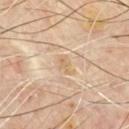A male patient aged 63–67. Located on the chest. Approximately 2.5 mm at its widest. Automated tile analysis of the lesion measured a mean CIELAB color near L≈68 a*≈15 b*≈35 and a lesion–skin lightness drop of about 6. The analysis additionally found a border-irregularity index near 3/10, internal color variation of about 2 on a 0–10 scale, and radial color variation of about 0.5. This is a cross-polarized tile. A 15 mm close-up extracted from a 3D total-body photography capture.